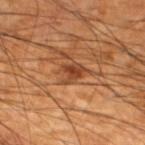biopsy status — imaged on a skin check; not biopsied | patient — male, roughly 60 years of age | anatomic site — the left lower leg | image — 15 mm crop, total-body photography.